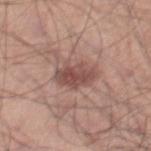Captured during whole-body skin photography for melanoma surveillance; the lesion was not biopsied.
A male subject in their mid- to late 40s.
On the leg.
A 15 mm close-up extracted from a 3D total-body photography capture.
The lesion-visualizer software estimated a mean CIELAB color near L≈49 a*≈21 b*≈23, roughly 11 lightness units darker than nearby skin, and a normalized lesion–skin contrast near 8. The software also gave border irregularity of about 2 on a 0–10 scale, internal color variation of about 4 on a 0–10 scale, and radial color variation of about 1.5. And it measured an automated nevus-likeness rating near 55 out of 100 and lesion-presence confidence of about 100/100.